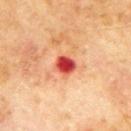The lesion was photographed on a routine skin check and not biopsied; there is no pathology result.
Imaged with cross-polarized lighting.
On the upper back.
A male subject in their 70s.
This image is a 15 mm lesion crop taken from a total-body photograph.